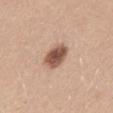Recorded during total-body skin imaging; not selected for excision or biopsy.
Located on the mid back.
The subject is a female aged approximately 25.
About 3.5 mm across.
A lesion tile, about 15 mm wide, cut from a 3D total-body photograph.
This is a white-light tile.